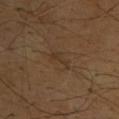The lesion is on the upper back. The patient is a male roughly 65 years of age. Cropped from a whole-body photographic skin survey; the tile spans about 15 mm.